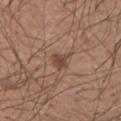Findings:
– anatomic site: the left upper arm
– subject: male, aged 53–57
– tile lighting: white-light
– image: ~15 mm crop, total-body skin-cancer survey